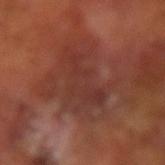Case summary:
• automated metrics: an automated nevus-likeness rating near 0 out of 100 and a detector confidence of about 85 out of 100 that the crop contains a lesion
• site: the arm
• lesion diameter: about 7.5 mm
• patient: male, aged 63 to 67
• lighting: cross-polarized
• image: total-body-photography crop, ~15 mm field of view